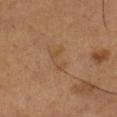Recorded during total-body skin imaging; not selected for excision or biopsy.
This is a cross-polarized tile.
Approximately 3.5 mm at its widest.
A 15 mm crop from a total-body photograph taken for skin-cancer surveillance.
From the right lower leg.
A male subject, aged around 55.